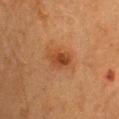  biopsy_status: not biopsied; imaged during a skin examination
  patient:
    sex: female
    age_approx: 60
  site: back
  image:
    source: total-body photography crop
    field_of_view_mm: 15